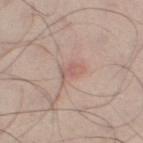Assessment:
Part of a total-body skin-imaging series; this lesion was reviewed on a skin check and was not flagged for biopsy.
Background:
A 15 mm close-up tile from a total-body photography series done for melanoma screening. The lesion is on the left thigh. The patient is a male aged 53 to 57. Longest diameter approximately 2.5 mm. The tile uses white-light illumination.